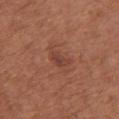Part of a total-body skin-imaging series; this lesion was reviewed on a skin check and was not flagged for biopsy.
This is a white-light tile.
The lesion is on the chest.
Longest diameter approximately 2.5 mm.
Automated image analysis of the tile measured a lesion color around L≈42 a*≈24 b*≈26 in CIELAB, a lesion–skin lightness drop of about 7, and a normalized border contrast of about 6.5.
A female subject roughly 65 years of age.
A lesion tile, about 15 mm wide, cut from a 3D total-body photograph.